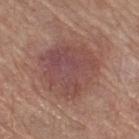Part of a total-body skin-imaging series; this lesion was reviewed on a skin check and was not flagged for biopsy.
A region of skin cropped from a whole-body photographic capture, roughly 15 mm wide.
Located on the right thigh.
The patient is a female about 65 years old.